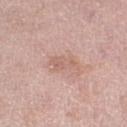biopsy status = total-body-photography surveillance lesion; no biopsy | site = the right lower leg | acquisition = 15 mm crop, total-body photography | patient = female, roughly 60 years of age | lighting = white-light | size = about 3.5 mm.